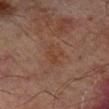Recorded during total-body skin imaging; not selected for excision or biopsy. Located on the left lower leg. The subject is a male aged 63 to 67. A 15 mm close-up extracted from a 3D total-body photography capture. This is a cross-polarized tile.